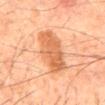{
  "biopsy_status": "not biopsied; imaged during a skin examination",
  "patient": {
    "sex": "male",
    "age_approx": 65
  },
  "site": "mid back",
  "lesion_size": {
    "long_diameter_mm_approx": 7.5
  },
  "lighting": "cross-polarized",
  "image": {
    "source": "total-body photography crop",
    "field_of_view_mm": 15
  },
  "automated_metrics": {
    "area_mm2_approx": 18.0,
    "eccentricity": 0.95,
    "shape_asymmetry": 0.15,
    "border_irregularity_0_10": 3.0,
    "color_variation_0_10": 3.5,
    "nevus_likeness_0_100": 65,
    "lesion_detection_confidence_0_100": 100
  }
}